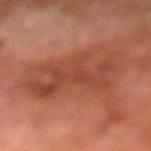| field | value |
|---|---|
| location | the left lower leg |
| tile lighting | cross-polarized illumination |
| subject | male, in their mid- to late 70s |
| size | about 7 mm |
| imaging modality | ~15 mm tile from a whole-body skin photo |
| image-analysis metrics | a footprint of about 24 mm², a shape eccentricity near 0.75, and a symmetry-axis asymmetry near 0.5; a color-variation rating of about 3/10 and a peripheral color-asymmetry measure near 1; a classifier nevus-likeness of about 0/100 and a lesion-detection confidence of about 100/100 |